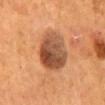Recorded during total-body skin imaging; not selected for excision or biopsy. Longest diameter approximately 5 mm. Imaged with cross-polarized lighting. On the mid back. A male subject roughly 55 years of age. A close-up tile cropped from a whole-body skin photograph, about 15 mm across.